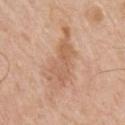Q: Is there a histopathology result?
A: total-body-photography surveillance lesion; no biopsy
Q: Lesion location?
A: the chest
Q: What kind of image is this?
A: ~15 mm crop, total-body skin-cancer survey
Q: What are the patient's age and sex?
A: male, aged 78 to 82
Q: How was the tile lit?
A: white-light